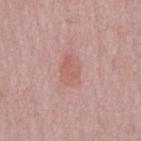<tbp_lesion>
  <biopsy_status>not biopsied; imaged during a skin examination</biopsy_status>
  <site>front of the torso</site>
  <patient>
    <sex>male</sex>
    <age_approx>50</age_approx>
  </patient>
  <image>
    <source>total-body photography crop</source>
    <field_of_view_mm>15</field_of_view_mm>
  </image>
  <automated_metrics>
    <area_mm2_approx>7.0</area_mm2_approx>
    <eccentricity>0.8</eccentricity>
    <shape_asymmetry>0.2</shape_asymmetry>
    <nevus_likeness_0_100>5</nevus_likeness_0_100>
    <lesion_detection_confidence_0_100>100</lesion_detection_confidence_0_100>
  </automated_metrics>
  <lesion_size>
    <long_diameter_mm_approx>3.5</long_diameter_mm_approx>
  </lesion_size>
</tbp_lesion>